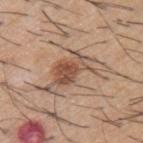{"biopsy_status": "not biopsied; imaged during a skin examination", "automated_metrics": {"area_mm2_approx": 16.0, "eccentricity": 0.9, "shape_asymmetry": 0.55, "cielab_L": 54, "cielab_a": 18, "cielab_b": 28, "vs_skin_darker_L": 11.0}, "lesion_size": {"long_diameter_mm_approx": 8.0}, "patient": {"sex": "male", "age_approx": 60}, "lighting": "white-light", "site": "upper back", "image": {"source": "total-body photography crop", "field_of_view_mm": 15}}